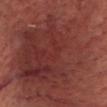Notes:
- notes: total-body-photography surveillance lesion; no biopsy
- tile lighting: cross-polarized
- body site: the head or neck
- automated lesion analysis: an area of roughly 175 mm² and two-axis asymmetry of about 0.35; an average lesion color of about L≈32 a*≈23 b*≈21 (CIELAB) and roughly 7 lightness units darker than nearby skin
- imaging modality: total-body-photography crop, ~15 mm field of view
- subject: male, approximately 60 years of age
- lesion size: ≈24.5 mm